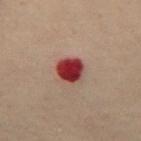Part of a total-body skin-imaging series; this lesion was reviewed on a skin check and was not flagged for biopsy.
Captured under cross-polarized illumination.
A roughly 15 mm field-of-view crop from a total-body skin photograph.
A male patient, aged 48–52.
Approximately 3.5 mm at its widest.
Located on the back.